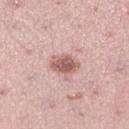Q: Was a biopsy performed?
A: imaged on a skin check; not biopsied
Q: Automated lesion metrics?
A: a lesion area of about 6 mm², an outline eccentricity of about 0.75 (0 = round, 1 = elongated), and a shape-asymmetry score of about 0.15 (0 = symmetric); a color-variation rating of about 3.5/10 and a peripheral color-asymmetry measure near 1; a classifier nevus-likeness of about 65/100 and lesion-presence confidence of about 100/100
Q: What is the lesion's diameter?
A: about 3.5 mm
Q: What is the anatomic site?
A: the right lower leg
Q: What is the imaging modality?
A: 15 mm crop, total-body photography
Q: Illumination type?
A: white-light
Q: What are the patient's age and sex?
A: female, in their mid-30s